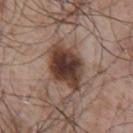Q: What kind of image is this?
A: ~15 mm crop, total-body skin-cancer survey
Q: Illumination type?
A: white-light illumination
Q: Patient demographics?
A: male, approximately 55 years of age
Q: What did automated image analysis measure?
A: a footprint of about 15 mm², an eccentricity of roughly 0.7, and a symmetry-axis asymmetry near 0.15; an average lesion color of about L≈37 a*≈18 b*≈23 (CIELAB), about 18 CIELAB-L* units darker than the surrounding skin, and a lesion-to-skin contrast of about 14 (normalized; higher = more distinct); border irregularity of about 2 on a 0–10 scale, a within-lesion color-variation index near 5.5/10, and radial color variation of about 1
Q: What is the anatomic site?
A: the chest